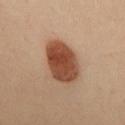The lesion was photographed on a routine skin check and not biopsied; there is no pathology result. Approximately 6 mm at its widest. A male patient, aged 43–47. An algorithmic analysis of the crop reported an area of roughly 17 mm² and a shape eccentricity near 0.8. And it measured an automated nevus-likeness rating near 100 out of 100 and a lesion-detection confidence of about 100/100. This image is a 15 mm lesion crop taken from a total-body photograph. This is a cross-polarized tile. On the mid back.